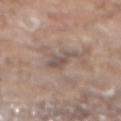lighting: white-light
image:
  source: total-body photography crop
  field_of_view_mm: 15
site: mid back
lesion_size:
  long_diameter_mm_approx: 3.0
patient:
  sex: male
  age_approx: 80